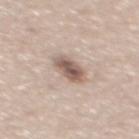Q: Was a biopsy performed?
A: catalogued during a skin exam; not biopsied
Q: Lesion size?
A: ≈3.5 mm
Q: Where on the body is the lesion?
A: the mid back
Q: What did automated image analysis measure?
A: about 14 CIELAB-L* units darker than the surrounding skin and a normalized border contrast of about 9; border irregularity of about 1.5 on a 0–10 scale, a within-lesion color-variation index near 5/10, and radial color variation of about 1.5
Q: What are the patient's age and sex?
A: male, aged approximately 45
Q: How was this image acquired?
A: ~15 mm crop, total-body skin-cancer survey